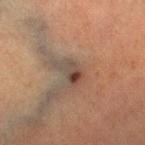<tbp_lesion>
<biopsy_status>not biopsied; imaged during a skin examination</biopsy_status>
<site>right lower leg</site>
<automated_metrics>
  <area_mm2_approx>5.0</area_mm2_approx>
  <eccentricity>0.8</eccentricity>
  <shape_asymmetry>0.3</shape_asymmetry>
  <vs_skin_darker_L>8.0</vs_skin_darker_L>
  <vs_skin_contrast_norm>7.5</vs_skin_contrast_norm>
  <border_irregularity_0_10>3.0</border_irregularity_0_10>
  <nevus_likeness_0_100>80</nevus_likeness_0_100>
  <lesion_detection_confidence_0_100>85</lesion_detection_confidence_0_100>
</automated_metrics>
<patient>
  <sex>female</sex>
  <age_approx>55</age_approx>
</patient>
<lesion_size>
  <long_diameter_mm_approx>3.0</long_diameter_mm_approx>
</lesion_size>
<image>
  <source>total-body photography crop</source>
  <field_of_view_mm>15</field_of_view_mm>
</image>
</tbp_lesion>